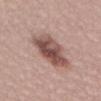Assessment:
Part of a total-body skin-imaging series; this lesion was reviewed on a skin check and was not flagged for biopsy.
Context:
A male subject aged around 50. Longest diameter approximately 5.5 mm. Captured under white-light illumination. The lesion is located on the lower back. Cropped from a total-body skin-imaging series; the visible field is about 15 mm.